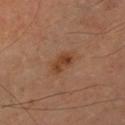workup: no biopsy performed (imaged during a skin exam) | image source: ~15 mm tile from a whole-body skin photo | patient: male, approximately 65 years of age | location: the left upper arm | automated lesion analysis: a footprint of about 5.5 mm², an outline eccentricity of about 0.8 (0 = round, 1 = elongated), and two-axis asymmetry of about 0.15; a lesion color around L≈42 a*≈22 b*≈33 in CIELAB, about 8 CIELAB-L* units darker than the surrounding skin, and a normalized lesion–skin contrast near 7.5 | size: ≈3 mm.